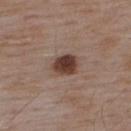This lesion was catalogued during total-body skin photography and was not selected for biopsy. Approximately 3 mm at its widest. The lesion is located on the upper back. A close-up tile cropped from a whole-body skin photograph, about 15 mm across. Imaged with white-light lighting. The total-body-photography lesion software estimated a footprint of about 7 mm², an eccentricity of roughly 0.55, and a shape-asymmetry score of about 0.15 (0 = symmetric). The analysis additionally found border irregularity of about 1.5 on a 0–10 scale, a within-lesion color-variation index near 5/10, and peripheral color asymmetry of about 1.5. It also reported an automated nevus-likeness rating near 100 out of 100 and lesion-presence confidence of about 100/100. A male patient aged approximately 55.